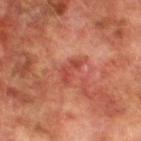This lesion was catalogued during total-body skin photography and was not selected for biopsy.
About 3 mm across.
A 15 mm close-up tile from a total-body photography series done for melanoma screening.
The lesion is on the leg.
The tile uses cross-polarized illumination.
A male patient about 75 years old.